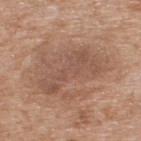Captured during whole-body skin photography for melanoma surveillance; the lesion was not biopsied.
From the upper back.
Longest diameter approximately 6.5 mm.
A male patient, aged approximately 60.
A close-up tile cropped from a whole-body skin photograph, about 15 mm across.
Automated tile analysis of the lesion measured an average lesion color of about L≈51 a*≈19 b*≈27 (CIELAB), about 7 CIELAB-L* units darker than the surrounding skin, and a lesion-to-skin contrast of about 5.5 (normalized; higher = more distinct).
The tile uses white-light illumination.